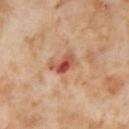This lesion was catalogued during total-body skin photography and was not selected for biopsy. Captured under cross-polarized illumination. Measured at roughly 3.5 mm in maximum diameter. On the right thigh. A 15 mm crop from a total-body photograph taken for skin-cancer surveillance. A female patient, aged 53–57.